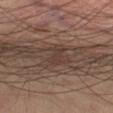Notes:
* follow-up — no biopsy performed (imaged during a skin exam)
* subject — male, in their mid-60s
* lesion size — ~2.5 mm (longest diameter)
* TBP lesion metrics — a lesion area of about 3 mm², an eccentricity of roughly 0.8, and two-axis asymmetry of about 0.45; a border-irregularity index near 4.5/10 and a within-lesion color-variation index near 0.5/10; an automated nevus-likeness rating near 0 out of 100 and a detector confidence of about 75 out of 100 that the crop contains a lesion
* anatomic site — the left thigh
* image — 15 mm crop, total-body photography
* tile lighting — cross-polarized illumination